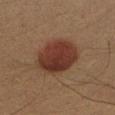biopsy status = no biopsy performed (imaged during a skin exam) | image = ~15 mm tile from a whole-body skin photo | subject = male, aged 38 to 42 | lesion size = ≈5 mm | image-analysis metrics = a lesion color around L≈31 a*≈20 b*≈24 in CIELAB and a normalized border contrast of about 10.5; a border-irregularity rating of about 1/10, a color-variation rating of about 4.5/10, and peripheral color asymmetry of about 1.5; lesion-presence confidence of about 100/100 | illumination = cross-polarized | body site = the right forearm.